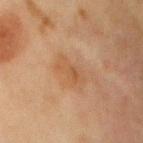| field | value |
|---|---|
| biopsy status | no biopsy performed (imaged during a skin exam) |